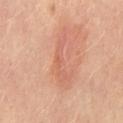Assessment:
No biopsy was performed on this lesion — it was imaged during a full skin examination and was not determined to be concerning.
Acquisition and patient details:
The recorded lesion diameter is about 6 mm. Located on the chest. A 15 mm close-up tile from a total-body photography series done for melanoma screening. The tile uses cross-polarized illumination. A female patient, aged approximately 50. An algorithmic analysis of the crop reported two-axis asymmetry of about 0.6. And it measured a classifier nevus-likeness of about 0/100 and lesion-presence confidence of about 85/100.